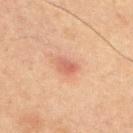Impression: Recorded during total-body skin imaging; not selected for excision or biopsy. Image and clinical context: A male subject aged 63–67. Captured under cross-polarized illumination. From the chest. About 2.5 mm across. This image is a 15 mm lesion crop taken from a total-body photograph.